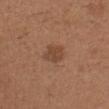Impression:
Recorded during total-body skin imaging; not selected for excision or biopsy.
Image and clinical context:
Automated tile analysis of the lesion measured a footprint of about 4.5 mm² and an eccentricity of roughly 0.65. It also reported border irregularity of about 2.5 on a 0–10 scale and a within-lesion color-variation index near 1.5/10. Captured under white-light illumination. Approximately 3 mm at its widest. The lesion is located on the left forearm. Cropped from a whole-body photographic skin survey; the tile spans about 15 mm. A male patient aged approximately 40.